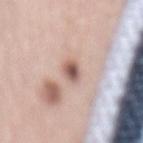<tbp_lesion>
<biopsy_status>not biopsied; imaged during a skin examination</biopsy_status>
</tbp_lesion>